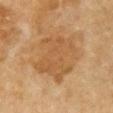No biopsy was performed on this lesion — it was imaged during a full skin examination and was not determined to be concerning.
This image is a 15 mm lesion crop taken from a total-body photograph.
From the chest.
The patient is a female in their mid-50s.